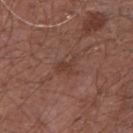Q: Was a biopsy performed?
A: catalogued during a skin exam; not biopsied
Q: What is the imaging modality?
A: total-body-photography crop, ~15 mm field of view
Q: Lesion location?
A: the chest
Q: Who is the patient?
A: male, aged 53–57
Q: How was the tile lit?
A: white-light
Q: Lesion size?
A: ~3 mm (longest diameter)